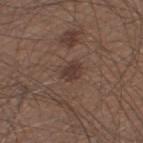notes: total-body-photography surveillance lesion; no biopsy | subject: male, roughly 45 years of age | location: the left thigh | image: ~15 mm tile from a whole-body skin photo | size: ~2.5 mm (longest diameter) | tile lighting: white-light illumination.